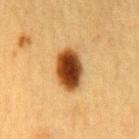Clinical impression: Part of a total-body skin-imaging series; this lesion was reviewed on a skin check and was not flagged for biopsy. Image and clinical context: This image is a 15 mm lesion crop taken from a total-body photograph. Measured at roughly 5 mm in maximum diameter. The lesion is on the mid back. Imaged with cross-polarized lighting. The patient is a female aged around 55.